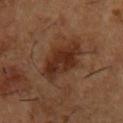A region of skin cropped from a whole-body photographic capture, roughly 15 mm wide.
Longest diameter approximately 6 mm.
On the chest.
The patient is a male in their mid-50s.
An algorithmic analysis of the crop reported a color-variation rating of about 4/10 and radial color variation of about 1.5. And it measured a lesion-detection confidence of about 100/100.
Captured under cross-polarized illumination.